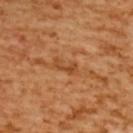| key | value |
|---|---|
| workup | imaged on a skin check; not biopsied |
| patient | female, aged approximately 55 |
| lesion diameter | about 3 mm |
| TBP lesion metrics | a shape eccentricity near 0.9; a lesion color around L≈48 a*≈26 b*≈42 in CIELAB, a lesion–skin lightness drop of about 9, and a normalized lesion–skin contrast near 6.5; a nevus-likeness score of about 0/100 |
| anatomic site | the upper back |
| illumination | cross-polarized illumination |
| image source | ~15 mm tile from a whole-body skin photo |